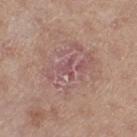Q: Is there a histopathology result?
A: catalogued during a skin exam; not biopsied